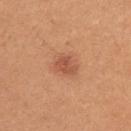Findings:
• biopsy status — no biopsy performed (imaged during a skin exam)
• lesion diameter — about 3 mm
• body site — the arm
• acquisition — ~15 mm tile from a whole-body skin photo
• patient — female, approximately 25 years of age
• automated metrics — an area of roughly 5 mm², an eccentricity of roughly 0.6, and a symmetry-axis asymmetry near 0.3; a mean CIELAB color near L≈54 a*≈27 b*≈34, roughly 10 lightness units darker than nearby skin, and a normalized border contrast of about 6.5; a nevus-likeness score of about 85/100 and a detector confidence of about 100 out of 100 that the crop contains a lesion